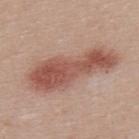Clinical impression: Part of a total-body skin-imaging series; this lesion was reviewed on a skin check and was not flagged for biopsy. Context: A male patient, aged 38 to 42. A 15 mm close-up extracted from a 3D total-body photography capture. On the upper back.